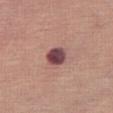Assessment:
The lesion was tiled from a total-body skin photograph and was not biopsied.
Acquisition and patient details:
The lesion is located on the leg. The subject is a female in their mid- to late 60s. Cropped from a whole-body photographic skin survey; the tile spans about 15 mm.